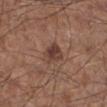Q: Was this lesion biopsied?
A: catalogued during a skin exam; not biopsied
Q: What is the imaging modality?
A: 15 mm crop, total-body photography
Q: What lighting was used for the tile?
A: white-light illumination
Q: Patient demographics?
A: male, roughly 60 years of age
Q: What is the lesion's diameter?
A: ~3 mm (longest diameter)
Q: Where on the body is the lesion?
A: the right lower leg